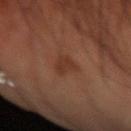This lesion was catalogued during total-body skin photography and was not selected for biopsy.
Longest diameter approximately 3 mm.
The lesion-visualizer software estimated a classifier nevus-likeness of about 40/100.
A lesion tile, about 15 mm wide, cut from a 3D total-body photograph.
This is a cross-polarized tile.
A male subject, roughly 70 years of age.
The lesion is on the right forearm.